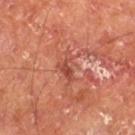Impression:
This lesion was catalogued during total-body skin photography and was not selected for biopsy.
Image and clinical context:
Measured at roughly 3.5 mm in maximum diameter. Located on the left thigh. Cropped from a whole-body photographic skin survey; the tile spans about 15 mm. Captured under cross-polarized illumination. A male patient, aged around 65.